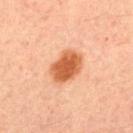Q: Was this lesion biopsied?
A: total-body-photography surveillance lesion; no biopsy